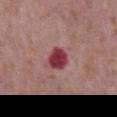Clinical impression:
Captured during whole-body skin photography for melanoma surveillance; the lesion was not biopsied.
Image and clinical context:
From the chest. Longest diameter approximately 3 mm. The patient is a male approximately 65 years of age. This image is a 15 mm lesion crop taken from a total-body photograph.